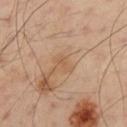Captured during whole-body skin photography for melanoma surveillance; the lesion was not biopsied. This is a cross-polarized tile. Automated tile analysis of the lesion measured an area of roughly 2.5 mm², a shape eccentricity near 0.9, and a shape-asymmetry score of about 0.4 (0 = symmetric). The analysis additionally found an automated nevus-likeness rating near 0 out of 100 and a lesion-detection confidence of about 100/100. Located on the left upper arm. Longest diameter approximately 2.5 mm. A male patient, about 50 years old. A region of skin cropped from a whole-body photographic capture, roughly 15 mm wide.